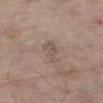biopsy status: catalogued during a skin exam; not biopsied | automated lesion analysis: border irregularity of about 3.5 on a 0–10 scale and a within-lesion color-variation index near 2/10; lesion-presence confidence of about 100/100 | subject: male, aged around 75 | illumination: white-light | image: 15 mm crop, total-body photography | location: the leg | lesion size: ~3 mm (longest diameter).